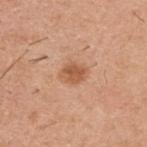Q: Is there a histopathology result?
A: no biopsy performed (imaged during a skin exam)
Q: Illumination type?
A: white-light illumination
Q: What is the anatomic site?
A: the back
Q: What is the imaging modality?
A: total-body-photography crop, ~15 mm field of view
Q: Who is the patient?
A: male, roughly 40 years of age
Q: Lesion size?
A: about 2.5 mm